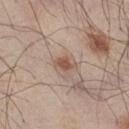Imaged during a routine full-body skin examination; the lesion was not biopsied and no histopathology is available. About 2.5 mm across. Automated tile analysis of the lesion measured a lesion color around L≈53 a*≈18 b*≈28 in CIELAB, about 11 CIELAB-L* units darker than the surrounding skin, and a normalized lesion–skin contrast near 8. The analysis additionally found a border-irregularity index near 2.5/10 and a peripheral color-asymmetry measure near 0.5. This is a white-light tile. On the right thigh. The subject is a male aged around 45. This image is a 15 mm lesion crop taken from a total-body photograph.